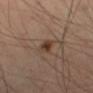Findings:
– workup — imaged on a skin check; not biopsied
– subject — male, aged approximately 65
– location — the right thigh
– image source — ~15 mm crop, total-body skin-cancer survey